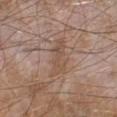Captured during whole-body skin photography for melanoma surveillance; the lesion was not biopsied.
Longest diameter approximately 4.5 mm.
A male patient in their mid- to late 60s.
Captured under white-light illumination.
A roughly 15 mm field-of-view crop from a total-body skin photograph.
The lesion is located on the left lower leg.